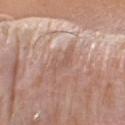{
  "biopsy_status": "not biopsied; imaged during a skin examination",
  "site": "arm",
  "image": {
    "source": "total-body photography crop",
    "field_of_view_mm": 15
  },
  "patient": {
    "sex": "male",
    "age_approx": 75
  }
}